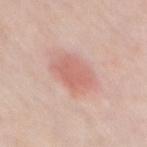Case summary:
- follow-up — total-body-photography surveillance lesion; no biopsy
- illumination — white-light illumination
- site — the chest
- subject — female, roughly 60 years of age
- lesion size — ~4 mm (longest diameter)
- image source — ~15 mm tile from a whole-body skin photo